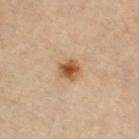The lesion was tiled from a total-body skin photograph and was not biopsied. The lesion is on the chest. A female subject, aged 28–32. The tile uses cross-polarized illumination. A 15 mm close-up tile from a total-body photography series done for melanoma screening. The recorded lesion diameter is about 2.5 mm. Automated tile analysis of the lesion measured border irregularity of about 1.5 on a 0–10 scale, internal color variation of about 4.5 on a 0–10 scale, and peripheral color asymmetry of about 1.